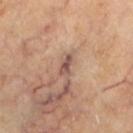Q: Was a biopsy performed?
A: total-body-photography surveillance lesion; no biopsy
Q: What are the patient's age and sex?
A: female, aged 58 to 62
Q: How large is the lesion?
A: ~2.5 mm (longest diameter)
Q: Where on the body is the lesion?
A: the leg
Q: Illumination type?
A: cross-polarized illumination
Q: What kind of image is this?
A: 15 mm crop, total-body photography